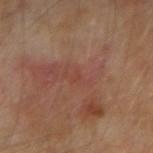biopsy status = no biopsy performed (imaged during a skin exam); site = the arm; lesion diameter = ≈1 mm; illumination = cross-polarized; image = 15 mm crop, total-body photography; subject = aged 53–57.